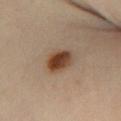  biopsy_status: not biopsied; imaged during a skin examination
  site: left lower leg
  patient:
    sex: female
    age_approx: 35
  image:
    source: total-body photography crop
    field_of_view_mm: 15
  lesion_size:
    long_diameter_mm_approx: 4.0